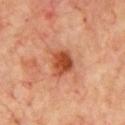Notes:
• follow-up · catalogued during a skin exam; not biopsied
• lesion size · about 3 mm
• image-analysis metrics · a footprint of about 7 mm², a shape eccentricity near 0.6, and two-axis asymmetry of about 0.2; an average lesion color of about L≈48 a*≈30 b*≈36 (CIELAB) and roughly 13 lightness units darker than nearby skin; a border-irregularity rating of about 1.5/10 and internal color variation of about 4.5 on a 0–10 scale
• body site · the chest
• patient · male, in their 70s
• tile lighting · cross-polarized illumination
• imaging modality · total-body-photography crop, ~15 mm field of view